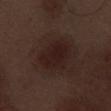The lesion was tiled from a total-body skin photograph and was not biopsied.
On the left thigh.
A male patient, about 70 years old.
A roughly 15 mm field-of-view crop from a total-body skin photograph.
Approximately 5 mm at its widest.
This is a white-light tile.